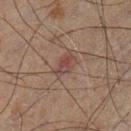The lesion was photographed on a routine skin check and not biopsied; there is no pathology result.
Captured under cross-polarized illumination.
Located on the left lower leg.
An algorithmic analysis of the crop reported a lesion area of about 4.5 mm², an eccentricity of roughly 0.8, and a shape-asymmetry score of about 0.2 (0 = symmetric). The software also gave a mean CIELAB color near L≈32 a*≈14 b*≈18, roughly 6 lightness units darker than nearby skin, and a normalized border contrast of about 6. It also reported a border-irregularity rating of about 2/10 and internal color variation of about 3 on a 0–10 scale. And it measured an automated nevus-likeness rating near 5 out of 100.
A male patient, aged 58–62.
A close-up tile cropped from a whole-body skin photograph, about 15 mm across.